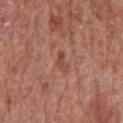Case summary:
• notes — no biopsy performed (imaged during a skin exam)
• location — the chest
• acquisition — total-body-photography crop, ~15 mm field of view
• lesion size — ~2.5 mm (longest diameter)
• subject — male, approximately 65 years of age